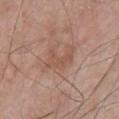image source = total-body-photography crop, ~15 mm field of view
subject = male, aged 53 to 57
body site = the chest